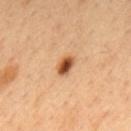<case>
<biopsy_status>not biopsied; imaged during a skin examination</biopsy_status>
<site>mid back</site>
<lesion_size>
  <long_diameter_mm_approx>2.5</long_diameter_mm_approx>
</lesion_size>
<image>
  <source>total-body photography crop</source>
  <field_of_view_mm>15</field_of_view_mm>
</image>
<patient>
  <sex>male</sex>
  <age_approx>50</age_approx>
</patient>
</case>